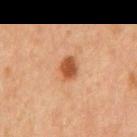{"site": "back", "patient": {"sex": "male", "age_approx": 65}, "image": {"source": "total-body photography crop", "field_of_view_mm": 15}}